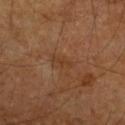Captured during whole-body skin photography for melanoma surveillance; the lesion was not biopsied.
Located on the arm.
A male patient, aged 58 to 62.
The tile uses cross-polarized illumination.
An algorithmic analysis of the crop reported a mean CIELAB color near L≈36 a*≈20 b*≈31, about 5 CIELAB-L* units darker than the surrounding skin, and a normalized border contrast of about 5. And it measured a border-irregularity rating of about 4.5/10, a within-lesion color-variation index near 0/10, and a peripheral color-asymmetry measure near 0. It also reported a nevus-likeness score of about 0/100.
A lesion tile, about 15 mm wide, cut from a 3D total-body photograph.
The lesion's longest dimension is about 2.5 mm.